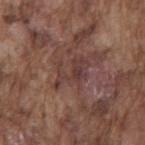| field | value |
|---|---|
| biopsy status | no biopsy performed (imaged during a skin exam) |
| tile lighting | white-light illumination |
| patient | male, aged 73–77 |
| body site | the mid back |
| image source | ~15 mm crop, total-body skin-cancer survey |